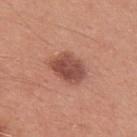notes — imaged on a skin check; not biopsied | lighting — white-light illumination | subject — male, roughly 30 years of age | size — about 4.5 mm | acquisition — ~15 mm tile from a whole-body skin photo | anatomic site — the left upper arm | automated metrics — an area of roughly 11 mm², an eccentricity of roughly 0.7, and two-axis asymmetry of about 0.2; a mean CIELAB color near L≈49 a*≈25 b*≈27, about 13 CIELAB-L* units darker than the surrounding skin, and a normalized lesion–skin contrast near 9; a color-variation rating of about 4/10 and peripheral color asymmetry of about 1.5; a nevus-likeness score of about 80/100 and lesion-presence confidence of about 100/100.